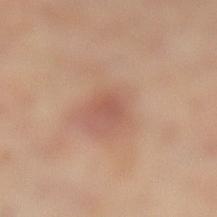{"biopsy_status": "not biopsied; imaged during a skin examination", "patient": {"sex": "female", "age_approx": 45}, "image": {"source": "total-body photography crop", "field_of_view_mm": 15}, "lesion_size": {"long_diameter_mm_approx": 2.0}, "site": "leg"}